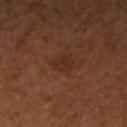notes: imaged on a skin check; not biopsied | lesion size: ~2.5 mm (longest diameter) | lighting: cross-polarized illumination | imaging modality: 15 mm crop, total-body photography | automated lesion analysis: a nevus-likeness score of about 30/100 and lesion-presence confidence of about 100/100 | patient: female, roughly 65 years of age | body site: the right upper arm.